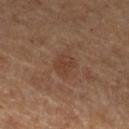Findings:
• biopsy status · catalogued during a skin exam; not biopsied
• illumination · cross-polarized illumination
• body site · the left lower leg
• diameter · ~3 mm (longest diameter)
• patient · male, aged around 65
• imaging modality · total-body-photography crop, ~15 mm field of view